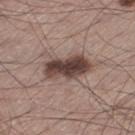| field | value |
|---|---|
| follow-up | total-body-photography surveillance lesion; no biopsy |
| subject | male, in their mid-50s |
| site | the leg |
| lesion size | ≈6 mm |
| image source | ~15 mm crop, total-body skin-cancer survey |